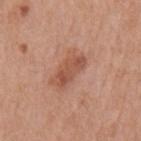<record>
<biopsy_status>not biopsied; imaged during a skin examination</biopsy_status>
<lighting>white-light</lighting>
<image>
  <source>total-body photography crop</source>
  <field_of_view_mm>15</field_of_view_mm>
</image>
<lesion_size>
  <long_diameter_mm_approx>4.0</long_diameter_mm_approx>
</lesion_size>
<site>mid back</site>
<patient>
  <sex>male</sex>
  <age_approx>70</age_approx>
</patient>
</record>